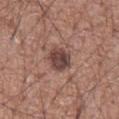Q: Is there a histopathology result?
A: total-body-photography surveillance lesion; no biopsy
Q: What did automated image analysis measure?
A: a peripheral color-asymmetry measure near 1.5
Q: Illumination type?
A: white-light illumination
Q: What are the patient's age and sex?
A: male, in their mid-70s
Q: What kind of image is this?
A: ~15 mm tile from a whole-body skin photo
Q: Lesion size?
A: ~3.5 mm (longest diameter)
Q: Where on the body is the lesion?
A: the abdomen